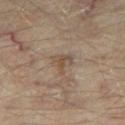Part of a total-body skin-imaging series; this lesion was reviewed on a skin check and was not flagged for biopsy.
The subject is about 55 years old.
The total-body-photography lesion software estimated a lesion area of about 4 mm² and an outline eccentricity of about 0.45 (0 = round, 1 = elongated). It also reported a lesion–skin lightness drop of about 7 and a normalized lesion–skin contrast near 6. The analysis additionally found a nevus-likeness score of about 0/100 and a detector confidence of about 100 out of 100 that the crop contains a lesion.
The lesion's longest dimension is about 2.5 mm.
A close-up tile cropped from a whole-body skin photograph, about 15 mm across.
On the right thigh.